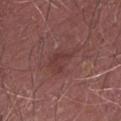Impression: Part of a total-body skin-imaging series; this lesion was reviewed on a skin check and was not flagged for biopsy. Clinical summary: From the right upper arm. A 15 mm close-up extracted from a 3D total-body photography capture. A male subject, roughly 70 years of age.